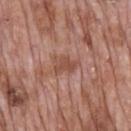subject — male, aged 68 to 72 | illumination — white-light illumination | acquisition — 15 mm crop, total-body photography | anatomic site — the mid back | lesion size — about 3 mm.